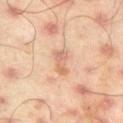No biopsy was performed on this lesion — it was imaged during a full skin examination and was not determined to be concerning.
The patient is a male in their mid-40s.
The lesion is on the right thigh.
A region of skin cropped from a whole-body photographic capture, roughly 15 mm wide.